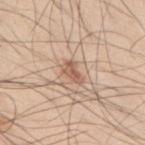Clinical impression:
Recorded during total-body skin imaging; not selected for excision or biopsy.
Image and clinical context:
Captured under white-light illumination. A male subject, about 45 years old. Automated tile analysis of the lesion measured a lesion color around L≈58 a*≈20 b*≈30 in CIELAB, roughly 11 lightness units darker than nearby skin, and a normalized lesion–skin contrast near 7.5. And it measured a border-irregularity index near 4/10. The analysis additionally found an automated nevus-likeness rating near 10 out of 100 and lesion-presence confidence of about 100/100. Longest diameter approximately 3 mm. On the upper back. Cropped from a whole-body photographic skin survey; the tile spans about 15 mm.